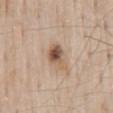Located on the mid back.
The patient is a male roughly 60 years of age.
A close-up tile cropped from a whole-body skin photograph, about 15 mm across.